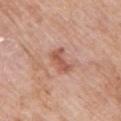<lesion>
<image>
  <source>total-body photography crop</source>
  <field_of_view_mm>15</field_of_view_mm>
</image>
<lesion_size>
  <long_diameter_mm_approx>3.0</long_diameter_mm_approx>
</lesion_size>
<lighting>white-light</lighting>
<site>arm</site>
<automated_metrics>
  <cielab_L>55</cielab_L>
  <cielab_a>25</cielab_a>
  <cielab_b>30</cielab_b>
  <vs_skin_contrast_norm>7.5</vs_skin_contrast_norm>
  <border_irregularity_0_10>4.5</border_irregularity_0_10>
  <color_variation_0_10>2.0</color_variation_0_10>
  <peripheral_color_asymmetry>0.5</peripheral_color_asymmetry>
</automated_metrics>
<patient>
  <sex>female</sex>
  <age_approx>70</age_approx>
</patient>
</lesion>